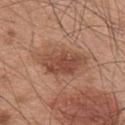  biopsy_status: not biopsied; imaged during a skin examination
  image:
    source: total-body photography crop
    field_of_view_mm: 15
  lighting: white-light
  site: upper back
  patient:
    sex: male
    age_approx: 45
  lesion_size:
    long_diameter_mm_approx: 6.0